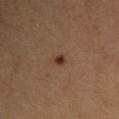Q: Is there a histopathology result?
A: no biopsy performed (imaged during a skin exam)
Q: Lesion size?
A: about 2 mm
Q: What is the imaging modality?
A: 15 mm crop, total-body photography
Q: Who is the patient?
A: female, aged approximately 35
Q: Where on the body is the lesion?
A: the right upper arm
Q: Automated lesion metrics?
A: a footprint of about 2.5 mm², an eccentricity of roughly 0.65, and two-axis asymmetry of about 0.2; a classifier nevus-likeness of about 95/100 and lesion-presence confidence of about 100/100